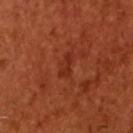biopsy status — no biopsy performed (imaged during a skin exam)
lesion diameter — about 2.5 mm
image — ~15 mm crop, total-body skin-cancer survey
subject — male, aged around 50
automated lesion analysis — a lesion area of about 2.5 mm², a shape eccentricity near 0.9, and two-axis asymmetry of about 0.45; a border-irregularity rating of about 5/10, a within-lesion color-variation index near 0/10, and a peripheral color-asymmetry measure near 0
anatomic site — the head or neck
tile lighting — cross-polarized